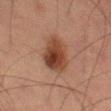workup: total-body-photography surveillance lesion; no biopsy | image source: ~15 mm crop, total-body skin-cancer survey | automated lesion analysis: an area of roughly 14 mm², an outline eccentricity of about 0.75 (0 = round, 1 = elongated), and two-axis asymmetry of about 0.15; a lesion color around L≈34 a*≈18 b*≈25 in CIELAB, roughly 11 lightness units darker than nearby skin, and a lesion-to-skin contrast of about 10 (normalized; higher = more distinct) | subject: male, aged 63 to 67 | illumination: cross-polarized illumination | anatomic site: the right thigh.